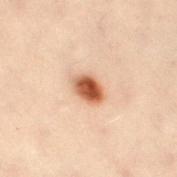follow-up — catalogued during a skin exam; not biopsied | patient — female, aged 28 to 32 | body site — the leg | image source — ~15 mm crop, total-body skin-cancer survey.